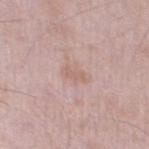Impression:
This lesion was catalogued during total-body skin photography and was not selected for biopsy.
Clinical summary:
Located on the left thigh. A male subject approximately 75 years of age. A lesion tile, about 15 mm wide, cut from a 3D total-body photograph. Imaged with white-light lighting.